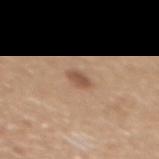The lesion was tiled from a total-body skin photograph and was not biopsied. A female subject, aged 53–57. An algorithmic analysis of the crop reported border irregularity of about 2 on a 0–10 scale and a peripheral color-asymmetry measure near 0.5. This image is a 15 mm lesion crop taken from a total-body photograph. Captured under white-light illumination. On the upper back. The recorded lesion diameter is about 2.5 mm.